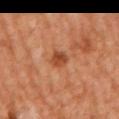Part of a total-body skin-imaging series; this lesion was reviewed on a skin check and was not flagged for biopsy. Measured at roughly 2.5 mm in maximum diameter. The subject is a male about 65 years old. Captured under cross-polarized illumination. A 15 mm close-up extracted from a 3D total-body photography capture. Located on the right upper arm.